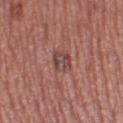  lesion_size:
    long_diameter_mm_approx: 3.5
  lighting: white-light
  site: left thigh
  patient:
    sex: female
    age_approx: 40
  image:
    source: total-body photography crop
    field_of_view_mm: 15